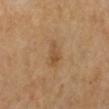Assessment: No biopsy was performed on this lesion — it was imaged during a full skin examination and was not determined to be concerning. Acquisition and patient details: Cropped from a whole-body photographic skin survey; the tile spans about 15 mm. A female patient, aged around 55. The lesion is located on the left lower leg.